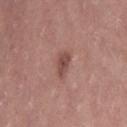Clinical summary:
Measured at roughly 3.5 mm in maximum diameter. Located on the left thigh. A region of skin cropped from a whole-body photographic capture, roughly 15 mm wide. The patient is a female aged 38 to 42.